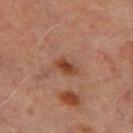biopsy_status: not biopsied; imaged during a skin examination
site: leg
lighting: cross-polarized
automated_metrics:
  lesion_detection_confidence_0_100: 100
image:
  source: total-body photography crop
  field_of_view_mm: 15
patient:
  sex: male
  age_approx: 70
lesion_size:
  long_diameter_mm_approx: 3.0